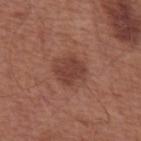| field | value |
|---|---|
| workup | total-body-photography surveillance lesion; no biopsy |
| lesion size | about 3.5 mm |
| body site | the abdomen |
| acquisition | ~15 mm crop, total-body skin-cancer survey |
| automated lesion analysis | an outline eccentricity of about 0.5 (0 = round, 1 = elongated); internal color variation of about 2 on a 0–10 scale and radial color variation of about 1 |
| patient | male, aged 63 to 67 |